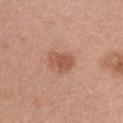biopsy_status: not biopsied; imaged during a skin examination
image:
  source: total-body photography crop
  field_of_view_mm: 15
patient:
  sex: female
  age_approx: 30
site: chest